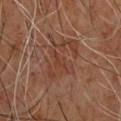No biopsy was performed on this lesion — it was imaged during a full skin examination and was not determined to be concerning. The patient is a male aged approximately 60. On the upper back. The recorded lesion diameter is about 6 mm. Imaged with cross-polarized lighting. Cropped from a total-body skin-imaging series; the visible field is about 15 mm.